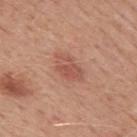Clinical impression:
The lesion was photographed on a routine skin check and not biopsied; there is no pathology result.
Acquisition and patient details:
This image is a 15 mm lesion crop taken from a total-body photograph. The lesion is on the upper back. Longest diameter approximately 4 mm. Imaged with white-light lighting. A male patient, about 50 years old. An algorithmic analysis of the crop reported a lesion color around L≈54 a*≈25 b*≈29 in CIELAB, about 8 CIELAB-L* units darker than the surrounding skin, and a normalized lesion–skin contrast near 6.